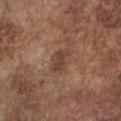This lesion was catalogued during total-body skin photography and was not selected for biopsy. Imaged with white-light lighting. The total-body-photography lesion software estimated an average lesion color of about L≈42 a*≈18 b*≈26 (CIELAB), a lesion–skin lightness drop of about 8, and a normalized lesion–skin contrast near 7. The analysis additionally found an automated nevus-likeness rating near 0 out of 100 and lesion-presence confidence of about 100/100. A lesion tile, about 15 mm wide, cut from a 3D total-body photograph. The patient is a male in their mid- to late 70s. Longest diameter approximately 3 mm. The lesion is on the chest.